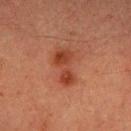The tile uses cross-polarized illumination. A roughly 15 mm field-of-view crop from a total-body skin photograph. The recorded lesion diameter is about 5 mm. Located on the right forearm. A female subject aged around 55.